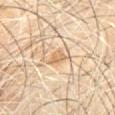biopsy status=no biopsy performed (imaged during a skin exam)
TBP lesion metrics=a border-irregularity rating of about 3.5/10
site=the back
acquisition=~15 mm tile from a whole-body skin photo
patient=male, aged 58 to 62
tile lighting=cross-polarized
lesion size=~3 mm (longest diameter)